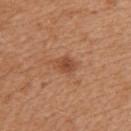{
  "automated_metrics": {
    "cielab_L": 49,
    "cielab_a": 24,
    "cielab_b": 34,
    "vs_skin_darker_L": 9.0,
    "vs_skin_contrast_norm": 6.5
  },
  "lighting": "white-light",
  "site": "left upper arm",
  "lesion_size": {
    "long_diameter_mm_approx": 3.0
  },
  "image": {
    "source": "total-body photography crop",
    "field_of_view_mm": 15
  },
  "patient": {
    "sex": "female",
    "age_approx": 40
  }
}